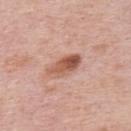Part of a total-body skin-imaging series; this lesion was reviewed on a skin check and was not flagged for biopsy. The subject is a male in their mid-70s. Located on the upper back. The tile uses white-light illumination. The recorded lesion diameter is about 4.5 mm. A region of skin cropped from a whole-body photographic capture, roughly 15 mm wide. Automated tile analysis of the lesion measured a mean CIELAB color near L≈56 a*≈24 b*≈30, a lesion–skin lightness drop of about 13, and a normalized border contrast of about 9. The software also gave an automated nevus-likeness rating near 90 out of 100 and lesion-presence confidence of about 100/100.